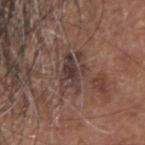This lesion was catalogued during total-body skin photography and was not selected for biopsy.
The lesion-visualizer software estimated a mean CIELAB color near L≈38 a*≈16 b*≈19, a lesion–skin lightness drop of about 9, and a normalized lesion–skin contrast near 8.5.
A male subject aged 68–72.
A 15 mm close-up tile from a total-body photography series done for melanoma screening.
The lesion's longest dimension is about 4.5 mm.
The tile uses white-light illumination.
The lesion is located on the head or neck.